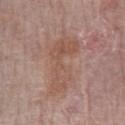Q: Is there a histopathology result?
A: catalogued during a skin exam; not biopsied
Q: Who is the patient?
A: female, aged 63–67
Q: How was this image acquired?
A: total-body-photography crop, ~15 mm field of view
Q: Lesion location?
A: the left forearm
Q: Automated lesion metrics?
A: roughly 6 lightness units darker than nearby skin and a normalized lesion–skin contrast near 5.5; border irregularity of about 5.5 on a 0–10 scale, a within-lesion color-variation index near 3.5/10, and a peripheral color-asymmetry measure near 1
Q: What is the lesion's diameter?
A: ≈7.5 mm